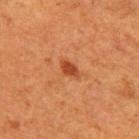The lesion was photographed on a routine skin check and not biopsied; there is no pathology result. On the right upper arm. A lesion tile, about 15 mm wide, cut from a 3D total-body photograph. The patient is a female aged 48 to 52.